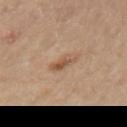The lesion was tiled from a total-body skin photograph and was not biopsied. The lesion is located on the right arm. This is a cross-polarized tile. The patient is a female aged around 60. Cropped from a total-body skin-imaging series; the visible field is about 15 mm. About 3 mm across.